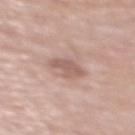  biopsy_status: not biopsied; imaged during a skin examination
  site: chest
  patient:
    sex: female
    age_approx: 65
  image:
    source: total-body photography crop
    field_of_view_mm: 15